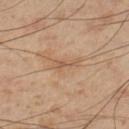Assessment:
Imaged during a routine full-body skin examination; the lesion was not biopsied and no histopathology is available.
Clinical summary:
A lesion tile, about 15 mm wide, cut from a 3D total-body photograph. The lesion is on the left lower leg. The subject is a male aged 53–57.